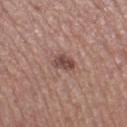biopsy_status: not biopsied; imaged during a skin examination
automated_metrics:
  cielab_L: 46
  cielab_a: 20
  cielab_b: 23
  vs_skin_darker_L: 11.0
  vs_skin_contrast_norm: 8.5
  border_irregularity_0_10: 2.5
  color_variation_0_10: 3.0
  peripheral_color_asymmetry: 1.0
  nevus_likeness_0_100: 60
  lesion_detection_confidence_0_100: 100
lesion_size:
  long_diameter_mm_approx: 2.5
site: left thigh
patient:
  sex: male
  age_approx: 75
image:
  source: total-body photography crop
  field_of_view_mm: 15